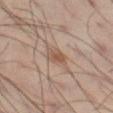Clinical impression: Imaged during a routine full-body skin examination; the lesion was not biopsied and no histopathology is available. Acquisition and patient details: A lesion tile, about 15 mm wide, cut from a 3D total-body photograph. Measured at roughly 2.5 mm in maximum diameter. Located on the leg. A male patient, aged 38 to 42. Imaged with cross-polarized lighting.